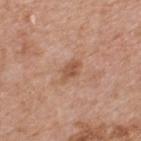subject = male, in their 60s; lesion size = ≈3 mm; anatomic site = the upper back; image = ~15 mm crop, total-body skin-cancer survey.